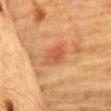The lesion was photographed on a routine skin check and not biopsied; there is no pathology result. A male subject in their mid- to late 80s or older. On the chest. The tile uses cross-polarized illumination. The lesion-visualizer software estimated a classifier nevus-likeness of about 0/100 and a lesion-detection confidence of about 100/100. A region of skin cropped from a whole-body photographic capture, roughly 15 mm wide.